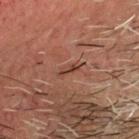Assessment: Recorded during total-body skin imaging; not selected for excision or biopsy. Clinical summary: The tile uses cross-polarized illumination. Approximately 3 mm at its widest. An algorithmic analysis of the crop reported an eccentricity of roughly 0.95. The software also gave a mean CIELAB color near L≈31 a*≈19 b*≈22, a lesion–skin lightness drop of about 7, and a normalized lesion–skin contrast near 7. And it measured a nevus-likeness score of about 0/100 and a detector confidence of about 60 out of 100 that the crop contains a lesion. On the head or neck. This image is a 15 mm lesion crop taken from a total-body photograph. The patient is a male about 65 years old.